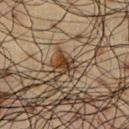Clinical summary:
A 15 mm crop from a total-body photograph taken for skin-cancer surveillance. The lesion is on the chest. A male subject, aged approximately 60.